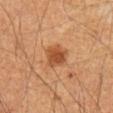Clinical summary:
Automated image analysis of the tile measured an area of roughly 7 mm², an outline eccentricity of about 0.4 (0 = round, 1 = elongated), and two-axis asymmetry of about 0.2. And it measured a mean CIELAB color near L≈45 a*≈23 b*≈35. And it measured a classifier nevus-likeness of about 95/100 and a lesion-detection confidence of about 100/100. This is a cross-polarized tile. About 3 mm across. A male patient roughly 60 years of age. A region of skin cropped from a whole-body photographic capture, roughly 15 mm wide. Located on the mid back.